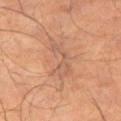Assessment: Imaged during a routine full-body skin examination; the lesion was not biopsied and no histopathology is available. Image and clinical context: The lesion is on the left thigh. A male subject, roughly 65 years of age. Cropped from a total-body skin-imaging series; the visible field is about 15 mm.